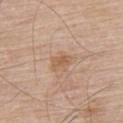notes: imaged on a skin check; not biopsied
anatomic site: the back
TBP lesion metrics: an area of roughly 3 mm², a shape eccentricity near 0.85, and a symmetry-axis asymmetry near 0.25
size: about 2.5 mm
lighting: white-light
patient: male, aged around 80
acquisition: ~15 mm crop, total-body skin-cancer survey